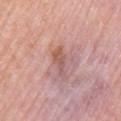Background: A region of skin cropped from a whole-body photographic capture, roughly 15 mm wide. The tile uses white-light illumination. The lesion is on the right upper arm. A female patient approximately 65 years of age. The total-body-photography lesion software estimated lesion-presence confidence of about 100/100.